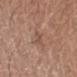workup: no biopsy performed (imaged during a skin exam) | illumination: white-light | subject: male, aged approximately 75 | lesion size: ~4 mm (longest diameter) | anatomic site: the left forearm | image: 15 mm crop, total-body photography.